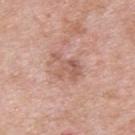biopsy status = catalogued during a skin exam; not biopsied | patient = male, approximately 40 years of age | anatomic site = the upper back | imaging modality = total-body-photography crop, ~15 mm field of view.